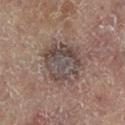workup: no biopsy performed (imaged during a skin exam) | image: ~15 mm tile from a whole-body skin photo | patient: female, approximately 80 years of age | site: the right leg | automated metrics: a footprint of about 18 mm², an eccentricity of roughly 0.4, and two-axis asymmetry of about 0.15; a mean CIELAB color near L≈40 a*≈11 b*≈16, roughly 8 lightness units darker than nearby skin, and a normalized border contrast of about 8; a border-irregularity rating of about 3/10, internal color variation of about 5.5 on a 0–10 scale, and peripheral color asymmetry of about 1.5 | tile lighting: cross-polarized illumination.